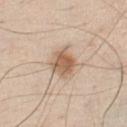Recorded during total-body skin imaging; not selected for excision or biopsy. The patient is a male roughly 45 years of age. A 15 mm crop from a total-body photograph taken for skin-cancer surveillance. The recorded lesion diameter is about 4 mm. The lesion is on the chest. An algorithmic analysis of the crop reported a mean CIELAB color near L≈61 a*≈17 b*≈31, roughly 12 lightness units darker than nearby skin, and a lesion-to-skin contrast of about 8 (normalized; higher = more distinct). It also reported a border-irregularity index near 2.5/10, internal color variation of about 5 on a 0–10 scale, and peripheral color asymmetry of about 1.5. This is a white-light tile.